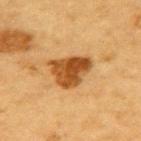No biopsy was performed on this lesion — it was imaged during a full skin examination and was not determined to be concerning. The lesion's longest dimension is about 5 mm. Imaged with cross-polarized lighting. The lesion is located on the upper back. A 15 mm close-up extracted from a 3D total-body photography capture. An algorithmic analysis of the crop reported a border-irregularity index near 3.5/10 and radial color variation of about 1.5. And it measured a nevus-likeness score of about 95/100. A male subject, roughly 85 years of age or older.